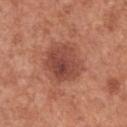This lesion was catalogued during total-body skin photography and was not selected for biopsy. The subject is a male aged 63–67. Measured at roughly 4.5 mm in maximum diameter. An algorithmic analysis of the crop reported a mean CIELAB color near L≈46 a*≈26 b*≈29, about 11 CIELAB-L* units darker than the surrounding skin, and a lesion-to-skin contrast of about 8 (normalized; higher = more distinct). And it measured a nevus-likeness score of about 80/100 and lesion-presence confidence of about 100/100. This is a white-light tile. The lesion is located on the abdomen. Cropped from a whole-body photographic skin survey; the tile spans about 15 mm.